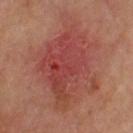This lesion was catalogued during total-body skin photography and was not selected for biopsy. On the left upper arm. Cropped from a total-body skin-imaging series; the visible field is about 15 mm. The lesion's longest dimension is about 10 mm. The patient is a male aged approximately 70. An algorithmic analysis of the crop reported border irregularity of about 5.5 on a 0–10 scale, a within-lesion color-variation index near 6.5/10, and peripheral color asymmetry of about 2. It also reported a detector confidence of about 100 out of 100 that the crop contains a lesion.